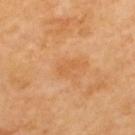follow-up = no biopsy performed (imaged during a skin exam) | image source = ~15 mm tile from a whole-body skin photo | site = the upper back | patient = male, in their 70s | image-analysis metrics = an average lesion color of about L≈58 a*≈24 b*≈42 (CIELAB) and a lesion–skin lightness drop of about 6; a border-irregularity rating of about 3.5/10, a within-lesion color-variation index near 0.5/10, and radial color variation of about 0 | illumination = cross-polarized illumination | lesion diameter = ≈3 mm.